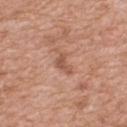<record>
  <image>
    <source>total-body photography crop</source>
    <field_of_view_mm>15</field_of_view_mm>
  </image>
  <lighting>white-light</lighting>
  <patient>
    <sex>male</sex>
    <age_approx>50</age_approx>
  </patient>
  <site>mid back</site>
  <automated_metrics>
    <area_mm2_approx>3.5</area_mm2_approx>
    <eccentricity>0.75</eccentricity>
    <cielab_L>54</cielab_L>
    <cielab_a>23</cielab_a>
    <cielab_b>30</cielab_b>
    <vs_skin_darker_L>9.0</vs_skin_darker_L>
    <vs_skin_contrast_norm>6.0</vs_skin_contrast_norm>
  </automated_metrics>
</record>